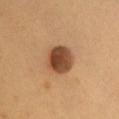<tbp_lesion>
<biopsy_status>not biopsied; imaged during a skin examination</biopsy_status>
<image>
  <source>total-body photography crop</source>
  <field_of_view_mm>15</field_of_view_mm>
</image>
<lighting>cross-polarized</lighting>
<patient>
  <sex>female</sex>
  <age_approx>35</age_approx>
</patient>
<site>front of the torso</site>
</tbp_lesion>